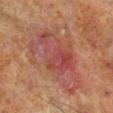Recorded during total-body skin imaging; not selected for excision or biopsy. Cropped from a whole-body photographic skin survey; the tile spans about 15 mm. The lesion's longest dimension is about 9 mm. The subject is a male aged around 70. Located on the right lower leg.